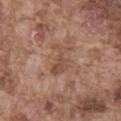Recorded during total-body skin imaging; not selected for excision or biopsy. Longest diameter approximately 4 mm. A lesion tile, about 15 mm wide, cut from a 3D total-body photograph. On the front of the torso. A male subject approximately 75 years of age. Imaged with white-light lighting.